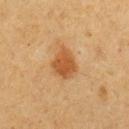Part of a total-body skin-imaging series; this lesion was reviewed on a skin check and was not flagged for biopsy.
About 4 mm across.
A lesion tile, about 15 mm wide, cut from a 3D total-body photograph.
A male subject, aged approximately 55.
On the chest.
Imaged with cross-polarized lighting.